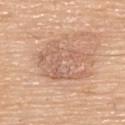The lesion was tiled from a total-body skin photograph and was not biopsied.
The total-body-photography lesion software estimated a shape eccentricity near 0.75 and a shape-asymmetry score of about 0.4 (0 = symmetric).
A male patient, about 80 years old.
Imaged with white-light lighting.
On the back.
A 15 mm close-up tile from a total-body photography series done for melanoma screening.